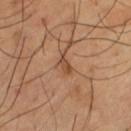Part of a total-body skin-imaging series; this lesion was reviewed on a skin check and was not flagged for biopsy. Located on the left thigh. Imaged with cross-polarized lighting. Cropped from a total-body skin-imaging series; the visible field is about 15 mm. A male patient, aged 63 to 67. Measured at roughly 2.5 mm in maximum diameter.